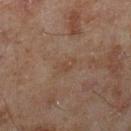Q: Was this lesion biopsied?
A: total-body-photography surveillance lesion; no biopsy
Q: Who is the patient?
A: male, approximately 45 years of age
Q: How was this image acquired?
A: total-body-photography crop, ~15 mm field of view
Q: How large is the lesion?
A: ~2.5 mm (longest diameter)
Q: Illumination type?
A: cross-polarized
Q: What is the anatomic site?
A: the right lower leg